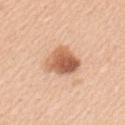| field | value |
|---|---|
| follow-up | catalogued during a skin exam; not biopsied |
| subject | female, aged around 45 |
| anatomic site | the left upper arm |
| tile lighting | white-light illumination |
| image source | ~15 mm tile from a whole-body skin photo |
| TBP lesion metrics | a footprint of about 11 mm² and an outline eccentricity of about 0.6 (0 = round, 1 = elongated); a lesion color around L≈60 a*≈24 b*≈34 in CIELAB, roughly 16 lightness units darker than nearby skin, and a normalized lesion–skin contrast near 9.5; a nevus-likeness score of about 95/100 and a detector confidence of about 100 out of 100 that the crop contains a lesion |